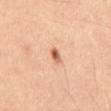This lesion was catalogued during total-body skin photography and was not selected for biopsy. From the abdomen. Approximately 2 mm at its widest. A 15 mm close-up tile from a total-body photography series done for melanoma screening. Automated tile analysis of the lesion measured roughly 14 lightness units darker than nearby skin and a normalized border contrast of about 9. A male patient, roughly 50 years of age.